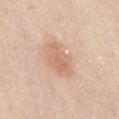Clinical impression:
Captured during whole-body skin photography for melanoma surveillance; the lesion was not biopsied.
Image and clinical context:
This is a white-light tile. The patient is a male about 65 years old. Approximately 4.5 mm at its widest. The lesion is located on the back. This image is a 15 mm lesion crop taken from a total-body photograph.